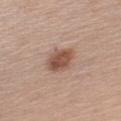A 15 mm close-up tile from a total-body photography series done for melanoma screening. A male subject, roughly 60 years of age. On the left upper arm.